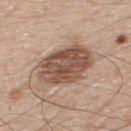Impression:
The lesion was tiled from a total-body skin photograph and was not biopsied.
Context:
Located on the upper back. The subject is a male aged 48 to 52. The recorded lesion diameter is about 6.5 mm. The total-body-photography lesion software estimated border irregularity of about 1.5 on a 0–10 scale and peripheral color asymmetry of about 2. A region of skin cropped from a whole-body photographic capture, roughly 15 mm wide.